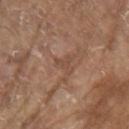Clinical impression: Recorded during total-body skin imaging; not selected for excision or biopsy. Image and clinical context: Longest diameter approximately 2.5 mm. A 15 mm close-up tile from a total-body photography series done for melanoma screening. A male subject, roughly 80 years of age. Captured under white-light illumination. On the right upper arm.